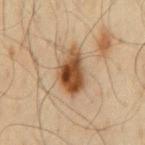The lesion was photographed on a routine skin check and not biopsied; there is no pathology result. Longest diameter approximately 5.5 mm. The tile uses cross-polarized illumination. This image is a 15 mm lesion crop taken from a total-body photograph. The subject is a male aged 63 to 67. On the mid back.